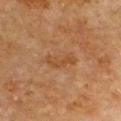follow-up = total-body-photography surveillance lesion; no biopsy.